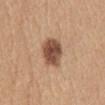This lesion was catalogued during total-body skin photography and was not selected for biopsy.
A close-up tile cropped from a whole-body skin photograph, about 15 mm across.
On the abdomen.
A female subject aged around 35.
Captured under white-light illumination.
About 4.5 mm across.